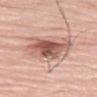The lesion was tiled from a total-body skin photograph and was not biopsied. The lesion is located on the left thigh. A male patient about 80 years old. A lesion tile, about 15 mm wide, cut from a 3D total-body photograph. About 8 mm across. Captured under white-light illumination.